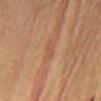The lesion was tiled from a total-body skin photograph and was not biopsied. A close-up tile cropped from a whole-body skin photograph, about 15 mm across. From the front of the torso. The lesion-visualizer software estimated an outline eccentricity of about 0.9 (0 = round, 1 = elongated) and a symmetry-axis asymmetry near 0.35. The software also gave a border-irregularity rating of about 4/10, a color-variation rating of about 0/10, and radial color variation of about 0. This is a cross-polarized tile. The lesion's longest dimension is about 2.5 mm. The patient is a female in their 60s.